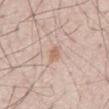Imaged during a routine full-body skin examination; the lesion was not biopsied and no histopathology is available.
The lesion-visualizer software estimated a footprint of about 3 mm². And it measured a nevus-likeness score of about 0/100 and a lesion-detection confidence of about 100/100.
The subject is a male aged 48–52.
This is a white-light tile.
From the back.
A region of skin cropped from a whole-body photographic capture, roughly 15 mm wide.